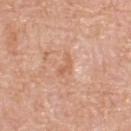This lesion was catalogued during total-body skin photography and was not selected for biopsy. A lesion tile, about 15 mm wide, cut from a 3D total-body photograph. Imaged with white-light lighting. The recorded lesion diameter is about 3 mm. A male subject aged 78–82. The lesion is located on the upper back.